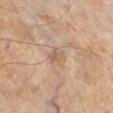Impression:
This lesion was catalogued during total-body skin photography and was not selected for biopsy.
Background:
From the right lower leg. A 15 mm close-up extracted from a 3D total-body photography capture. The patient is a male aged 63 to 67. This is a cross-polarized tile.